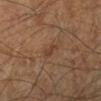biopsy_status: not biopsied; imaged during a skin examination
lesion_size:
  long_diameter_mm_approx: 2.5
automated_metrics:
  area_mm2_approx: 2.5
  shape_asymmetry: 0.4
patient:
  sex: male
  age_approx: 60
image:
  source: total-body photography crop
  field_of_view_mm: 15
site: left lower leg
lighting: cross-polarized